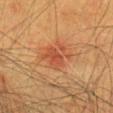{"site": "head or neck", "patient": {"sex": "female", "age_approx": 55}, "lesion_size": {"long_diameter_mm_approx": 4.0}, "image": {"source": "total-body photography crop", "field_of_view_mm": 15}, "lighting": "cross-polarized"}